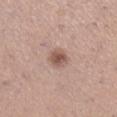notes — imaged on a skin check; not biopsied
automated lesion analysis — a footprint of about 4.5 mm², an eccentricity of roughly 0.55, and a symmetry-axis asymmetry near 0.2; a lesion color around L≈54 a*≈20 b*≈25 in CIELAB and about 12 CIELAB-L* units darker than the surrounding skin; a border-irregularity index near 1.5/10 and a peripheral color-asymmetry measure near 1; a classifier nevus-likeness of about 90/100 and lesion-presence confidence of about 100/100
tile lighting — white-light
patient — female, aged 28 to 32
acquisition — total-body-photography crop, ~15 mm field of view
anatomic site — the right lower leg